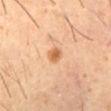Case summary:
* biopsy status: imaged on a skin check; not biopsied
* location: the abdomen
* lesion diameter: ~2 mm (longest diameter)
* acquisition: ~15 mm tile from a whole-body skin photo
* TBP lesion metrics: border irregularity of about 2 on a 0–10 scale, a within-lesion color-variation index near 3/10, and a peripheral color-asymmetry measure near 1; an automated nevus-likeness rating near 80 out of 100
* subject: male, aged 58 to 62